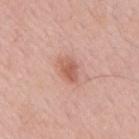No biopsy was performed on this lesion — it was imaged during a full skin examination and was not determined to be concerning. About 3 mm across. A 15 mm crop from a total-body photograph taken for skin-cancer surveillance. A male subject about 55 years old. The lesion is located on the mid back. The total-body-photography lesion software estimated a footprint of about 4.5 mm², an eccentricity of roughly 0.75, and two-axis asymmetry of about 0.2. And it measured about 10 CIELAB-L* units darker than the surrounding skin. The software also gave a nevus-likeness score of about 75/100 and lesion-presence confidence of about 100/100.